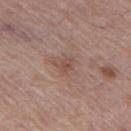The lesion was tiled from a total-body skin photograph and was not biopsied. A lesion tile, about 15 mm wide, cut from a 3D total-body photograph. From the left thigh. Longest diameter approximately 2.5 mm. A female subject aged 63–67. Imaged with white-light lighting.